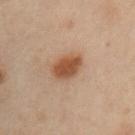The lesion was photographed on a routine skin check and not biopsied; there is no pathology result. Measured at roughly 3.5 mm in maximum diameter. On the right upper arm. The patient is a male approximately 55 years of age. Imaged with cross-polarized lighting. A 15 mm crop from a total-body photograph taken for skin-cancer surveillance.